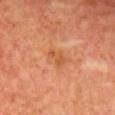Recorded during total-body skin imaging; not selected for excision or biopsy. A female subject aged 53–57. Cropped from a whole-body photographic skin survey; the tile spans about 15 mm. The lesion is located on the front of the torso.